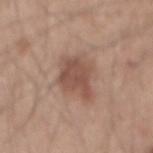Imaged during a routine full-body skin examination; the lesion was not biopsied and no histopathology is available.
A male patient, in their mid- to late 40s.
A close-up tile cropped from a whole-body skin photograph, about 15 mm across.
The total-body-photography lesion software estimated roughly 10 lightness units darker than nearby skin and a normalized border contrast of about 7.5. The software also gave a border-irregularity index near 3/10, a color-variation rating of about 2.5/10, and peripheral color asymmetry of about 1.
From the mid back.